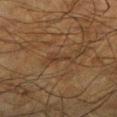Part of a total-body skin-imaging series; this lesion was reviewed on a skin check and was not flagged for biopsy.
Automated image analysis of the tile measured a footprint of about 4 mm², an eccentricity of roughly 0.9, and a symmetry-axis asymmetry near 0.55. It also reported a border-irregularity rating of about 6.5/10 and a within-lesion color-variation index near 2/10. The analysis additionally found a classifier nevus-likeness of about 0/100 and a lesion-detection confidence of about 55/100.
The subject is a male aged 63 to 67.
The tile uses cross-polarized illumination.
From the left lower leg.
Measured at roughly 3.5 mm in maximum diameter.
Cropped from a whole-body photographic skin survey; the tile spans about 15 mm.